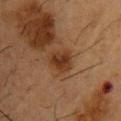Q: Is there a histopathology result?
A: imaged on a skin check; not biopsied
Q: Lesion location?
A: the upper back
Q: What are the patient's age and sex?
A: male, approximately 55 years of age
Q: What is the imaging modality?
A: total-body-photography crop, ~15 mm field of view